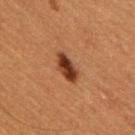The lesion was photographed on a routine skin check and not biopsied; there is no pathology result. The subject is a male in their 50s. From the right upper arm. This image is a 15 mm lesion crop taken from a total-body photograph. Measured at roughly 3 mm in maximum diameter. This is a cross-polarized tile.